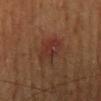Part of a total-body skin-imaging series; this lesion was reviewed on a skin check and was not flagged for biopsy.
A male subject, aged around 60.
The lesion's longest dimension is about 4 mm.
Automated tile analysis of the lesion measured an outline eccentricity of about 0.7 (0 = round, 1 = elongated) and two-axis asymmetry of about 0.2. It also reported a border-irregularity index near 2.5/10 and radial color variation of about 1.5. The analysis additionally found a nevus-likeness score of about 40/100.
From the mid back.
Cropped from a total-body skin-imaging series; the visible field is about 15 mm.
The tile uses cross-polarized illumination.